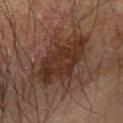| key | value |
|---|---|
| site | the arm |
| lesion size | about 7.5 mm |
| subject | male, in their 70s |
| image | ~15 mm crop, total-body skin-cancer survey |
| lighting | cross-polarized |